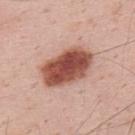Q: Was a biopsy performed?
A: total-body-photography surveillance lesion; no biopsy
Q: What kind of image is this?
A: 15 mm crop, total-body photography
Q: Lesion location?
A: the upper back
Q: How was the tile lit?
A: white-light illumination
Q: Automated lesion metrics?
A: an outline eccentricity of about 0.8 (0 = round, 1 = elongated) and two-axis asymmetry of about 0.15
Q: Patient demographics?
A: male, aged 33–37
Q: Lesion size?
A: ~6.5 mm (longest diameter)